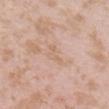biopsy_status: not biopsied; imaged during a skin examination